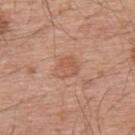notes = imaged on a skin check; not biopsied
body site = the upper back
diameter = about 3 mm
subject = male, aged around 55
acquisition = total-body-photography crop, ~15 mm field of view